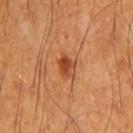| feature | finding |
|---|---|
| lesion size | about 2.5 mm |
| lighting | cross-polarized |
| acquisition | 15 mm crop, total-body photography |
| location | the arm |
| subject | male, aged approximately 60 |